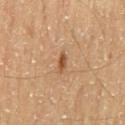{"biopsy_status": "not biopsied; imaged during a skin examination", "lighting": "cross-polarized", "image": {"source": "total-body photography crop", "field_of_view_mm": 15}, "patient": {"sex": "male", "age_approx": 55}, "site": "mid back", "automated_metrics": {"cielab_L": 50, "cielab_a": 22, "cielab_b": 36, "vs_skin_contrast_norm": 8.5}, "lesion_size": {"long_diameter_mm_approx": 2.5}}